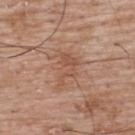Assessment: No biopsy was performed on this lesion — it was imaged during a full skin examination and was not determined to be concerning. Context: Located on the upper back. This image is a 15 mm lesion crop taken from a total-body photograph. A male subject, aged 48–52. Imaged with white-light lighting.